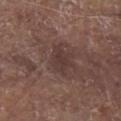Q: Was this lesion biopsied?
A: total-body-photography surveillance lesion; no biopsy
Q: Patient demographics?
A: male, aged 78 to 82
Q: What is the imaging modality?
A: total-body-photography crop, ~15 mm field of view
Q: How was the tile lit?
A: white-light illumination
Q: Lesion location?
A: the chest